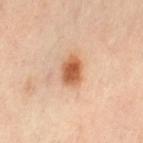Q: Was this lesion biopsied?
A: no biopsy performed (imaged during a skin exam)
Q: Patient demographics?
A: female, about 40 years old
Q: Lesion size?
A: ≈3.5 mm
Q: Illumination type?
A: cross-polarized illumination
Q: Automated lesion metrics?
A: an area of roughly 7 mm², a shape eccentricity near 0.7, and a symmetry-axis asymmetry near 0.15
Q: What is the imaging modality?
A: ~15 mm tile from a whole-body skin photo
Q: Lesion location?
A: the leg